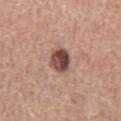This lesion was catalogued during total-body skin photography and was not selected for biopsy. On the abdomen. Longest diameter approximately 3.5 mm. A region of skin cropped from a whole-body photographic capture, roughly 15 mm wide. Imaged with white-light lighting. The subject is a male aged 73–77.